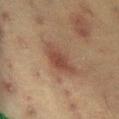Case summary:
- follow-up · no biopsy performed (imaged during a skin exam)
- body site · the abdomen
- patient · female, aged 38 to 42
- lesion diameter · ≈5 mm
- tile lighting · cross-polarized illumination
- image source · 15 mm crop, total-body photography